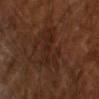patient = male, in their mid- to late 60s; image = ~15 mm tile from a whole-body skin photo.